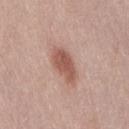{
  "biopsy_status": "not biopsied; imaged during a skin examination",
  "patient": {
    "sex": "female",
    "age_approx": 40
  },
  "image": {
    "source": "total-body photography crop",
    "field_of_view_mm": 15
  },
  "site": "lower back",
  "lighting": "white-light",
  "automated_metrics": {
    "cielab_L": 55,
    "cielab_a": 22,
    "cielab_b": 27,
    "vs_skin_darker_L": 12.0,
    "vs_skin_contrast_norm": 8.5,
    "border_irregularity_0_10": 2.0,
    "color_variation_0_10": 2.5,
    "peripheral_color_asymmetry": 1.0,
    "nevus_likeness_0_100": 95,
    "lesion_detection_confidence_0_100": 100
  },
  "lesion_size": {
    "long_diameter_mm_approx": 4.5
  }
}